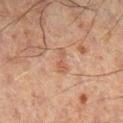Assessment:
Captured during whole-body skin photography for melanoma surveillance; the lesion was not biopsied.
Context:
The tile uses cross-polarized illumination. On the leg. Automated tile analysis of the lesion measured an eccentricity of roughly 0.95 and a symmetry-axis asymmetry near 0.45. The analysis additionally found roughly 5 lightness units darker than nearby skin and a lesion-to-skin contrast of about 5 (normalized; higher = more distinct). The analysis additionally found a border-irregularity index near 5.5/10, a color-variation rating of about 0/10, and a peripheral color-asymmetry measure near 0. The software also gave a nevus-likeness score of about 0/100. Measured at roughly 3 mm in maximum diameter. A 15 mm close-up tile from a total-body photography series done for melanoma screening. The patient is a male aged 58 to 62.